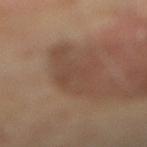{"biopsy_status": "not biopsied; imaged during a skin examination", "lesion_size": {"long_diameter_mm_approx": 7.5}, "patient": {"sex": "female", "age_approx": 65}, "image": {"source": "total-body photography crop", "field_of_view_mm": 15}, "lighting": "cross-polarized", "site": "left leg"}